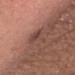biopsy status = catalogued during a skin exam; not biopsied | site = the head or neck | patient = male, aged approximately 65 | lighting = white-light | lesion size = about 4.5 mm | image source = 15 mm crop, total-body photography.